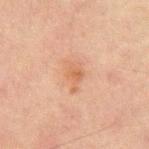follow-up = total-body-photography surveillance lesion; no biopsy
size = ~3.5 mm (longest diameter)
acquisition = ~15 mm tile from a whole-body skin photo
patient = male, in their mid- to late 60s
image-analysis metrics = an automated nevus-likeness rating near 10 out of 100 and a lesion-detection confidence of about 100/100
tile lighting = cross-polarized
site = the mid back